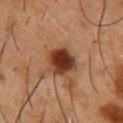Clinical summary:
The lesion is on the chest. An algorithmic analysis of the crop reported a lesion–skin lightness drop of about 17 and a normalized lesion–skin contrast near 13. The software also gave an automated nevus-likeness rating near 100 out of 100 and a detector confidence of about 100 out of 100 that the crop contains a lesion. Approximately 3.5 mm at its widest. Cropped from a total-body skin-imaging series; the visible field is about 15 mm. The subject is a male in their mid-50s.